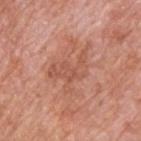workup: no biopsy performed (imaged during a skin exam)
lighting: white-light illumination
image-analysis metrics: an average lesion color of about L≈55 a*≈26 b*≈31 (CIELAB), roughly 7 lightness units darker than nearby skin, and a lesion-to-skin contrast of about 5 (normalized; higher = more distinct)
body site: the back
image: ~15 mm crop, total-body skin-cancer survey
patient: male, about 60 years old